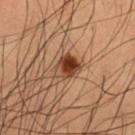Impression:
Part of a total-body skin-imaging series; this lesion was reviewed on a skin check and was not flagged for biopsy.
Background:
A male patient aged approximately 50. Located on the left thigh. The tile uses cross-polarized illumination. Measured at roughly 3.5 mm in maximum diameter. This image is a 15 mm lesion crop taken from a total-body photograph.